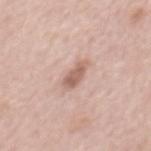workup — catalogued during a skin exam; not biopsied | subject — male, aged 53–57 | image source — ~15 mm tile from a whole-body skin photo | location — the mid back | lighting — white-light.